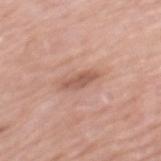notes = no biopsy performed (imaged during a skin exam)